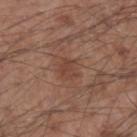Q: Was a biopsy performed?
A: imaged on a skin check; not biopsied
Q: How was this image acquired?
A: ~15 mm tile from a whole-body skin photo
Q: Who is the patient?
A: male, about 55 years old
Q: Lesion location?
A: the left forearm